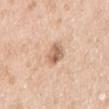The lesion was photographed on a routine skin check and not biopsied; there is no pathology result. The lesion is located on the right upper arm. The patient is a female aged approximately 50. Captured under white-light illumination. The lesion-visualizer software estimated an area of roughly 7.5 mm², an outline eccentricity of about 0.35 (0 = round, 1 = elongated), and a symmetry-axis asymmetry near 0.25. The lesion's longest dimension is about 3 mm. A 15 mm close-up extracted from a 3D total-body photography capture.